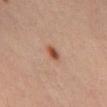| feature | finding |
|---|---|
| workup | total-body-photography surveillance lesion; no biopsy |
| patient | male, about 65 years old |
| image | 15 mm crop, total-body photography |
| site | the mid back |